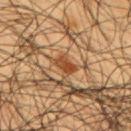* biopsy status — no biopsy performed (imaged during a skin exam)
* size — ~2.5 mm (longest diameter)
* subject — male, roughly 60 years of age
* lighting — cross-polarized
* body site — the upper back
* image — 15 mm crop, total-body photography